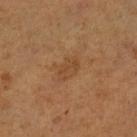Notes:
– biopsy status · imaged on a skin check; not biopsied
– subject · male, aged around 55
– image source · ~15 mm tile from a whole-body skin photo
– anatomic site · the left lower leg
– tile lighting · cross-polarized
– diameter · about 3.5 mm